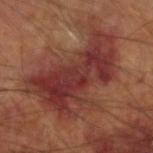No biopsy was performed on this lesion — it was imaged during a full skin examination and was not determined to be concerning.
About 10.5 mm across.
The lesion is located on the left arm.
This image is a 15 mm lesion crop taken from a total-body photograph.
A male subject, roughly 65 years of age.
Imaged with cross-polarized lighting.